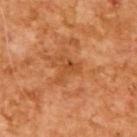Clinical impression:
Captured during whole-body skin photography for melanoma surveillance; the lesion was not biopsied.
Context:
Automated image analysis of the tile measured a lesion color around L≈48 a*≈27 b*≈41 in CIELAB and a lesion–skin lightness drop of about 7. It also reported a classifier nevus-likeness of about 0/100 and lesion-presence confidence of about 100/100. Longest diameter approximately 3 mm. A male patient aged 63–67. Captured under cross-polarized illumination. A 15 mm close-up tile from a total-body photography series done for melanoma screening.